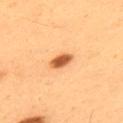A male patient in their mid-50s. A 15 mm close-up extracted from a 3D total-body photography capture. This is a cross-polarized tile. From the upper back. An algorithmic analysis of the crop reported a footprint of about 4 mm² and a shape-asymmetry score of about 0.2 (0 = symmetric).